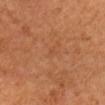Recorded during total-body skin imaging; not selected for excision or biopsy. A close-up tile cropped from a whole-body skin photograph, about 15 mm across. A female patient, roughly 45 years of age. Located on the head or neck. This is a cross-polarized tile.